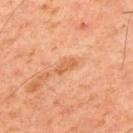– follow-up — catalogued during a skin exam; not biopsied
– location — the upper back
– subject — male, aged 58–62
– illumination — cross-polarized illumination
– automated lesion analysis — an area of roughly 3 mm², a shape eccentricity near 0.85, and two-axis asymmetry of about 0.3; a border-irregularity index near 2.5/10, a within-lesion color-variation index near 1/10, and radial color variation of about 0; a nevus-likeness score of about 0/100 and a lesion-detection confidence of about 100/100
– image source — total-body-photography crop, ~15 mm field of view
– size — about 2.5 mm